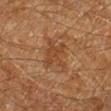Clinical impression:
The lesion was tiled from a total-body skin photograph and was not biopsied.
Background:
Located on the left lower leg. A male subject aged approximately 60. An algorithmic analysis of the crop reported a lesion color around L≈33 a*≈17 b*≈28 in CIELAB, about 6 CIELAB-L* units darker than the surrounding skin, and a normalized lesion–skin contrast near 5.5. The analysis additionally found a border-irregularity index near 5.5/10, internal color variation of about 1.5 on a 0–10 scale, and peripheral color asymmetry of about 0.5. A 15 mm crop from a total-body photograph taken for skin-cancer surveillance. The tile uses cross-polarized illumination. Measured at roughly 4.5 mm in maximum diameter.